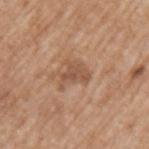biopsy status = catalogued during a skin exam; not biopsied | acquisition = ~15 mm crop, total-body skin-cancer survey | patient = male, in their 60s | anatomic site = the left upper arm | size = about 3.5 mm | lighting = white-light illumination.